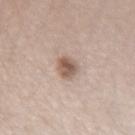workup = no biopsy performed (imaged during a skin exam) | site = the right forearm | subject = female, approximately 40 years of age | lighting = white-light illumination | imaging modality = total-body-photography crop, ~15 mm field of view.